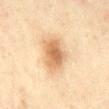Clinical impression:
Imaged during a routine full-body skin examination; the lesion was not biopsied and no histopathology is available.
Acquisition and patient details:
The lesion-visualizer software estimated a lesion color around L≈62 a*≈17 b*≈35 in CIELAB, about 12 CIELAB-L* units darker than the surrounding skin, and a normalized border contrast of about 7.5. And it measured border irregularity of about 2 on a 0–10 scale, internal color variation of about 5 on a 0–10 scale, and peripheral color asymmetry of about 1. The software also gave an automated nevus-likeness rating near 95 out of 100 and a lesion-detection confidence of about 100/100. A roughly 15 mm field-of-view crop from a total-body skin photograph. From the mid back. A female patient aged approximately 60.